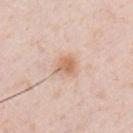Q: Was this lesion biopsied?
A: no biopsy performed (imaged during a skin exam)
Q: What are the patient's age and sex?
A: male, approximately 35 years of age
Q: Illumination type?
A: white-light
Q: Lesion location?
A: the chest
Q: What is the lesion's diameter?
A: about 2.5 mm
Q: What is the imaging modality?
A: total-body-photography crop, ~15 mm field of view
Q: Automated lesion metrics?
A: a mean CIELAB color near L≈66 a*≈19 b*≈31 and a normalized lesion–skin contrast near 7; a border-irregularity index near 2/10, a within-lesion color-variation index near 4/10, and radial color variation of about 1.5; a classifier nevus-likeness of about 70/100